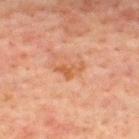<case>
  <biopsy_status>not biopsied; imaged during a skin examination</biopsy_status>
  <lesion_size>
    <long_diameter_mm_approx>3.5</long_diameter_mm_approx>
  </lesion_size>
  <site>upper back</site>
  <patient>
    <sex>male</sex>
    <age_approx>60</age_approx>
  </patient>
  <automated_metrics>
    <lesion_detection_confidence_0_100>100</lesion_detection_confidence_0_100>
  </automated_metrics>
  <image>
    <source>total-body photography crop</source>
    <field_of_view_mm>15</field_of_view_mm>
  </image>
  <lighting>cross-polarized</lighting>
</case>